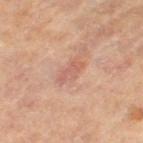  biopsy_status: not biopsied; imaged during a skin examination
  image:
    source: total-body photography crop
    field_of_view_mm: 15
  site: right thigh
  lesion_size:
    long_diameter_mm_approx: 3.5
  patient:
    sex: male
    age_approx: 85+
  lighting: cross-polarized